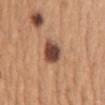site: the abdomen
size: ≈3.5 mm
acquisition: ~15 mm tile from a whole-body skin photo
tile lighting: white-light
subject: female, aged 33–37